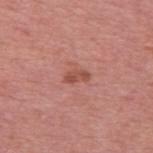biopsy status=imaged on a skin check; not biopsied
lesion diameter=≈2.5 mm
imaging modality=~15 mm tile from a whole-body skin photo
patient=male, approximately 55 years of age
anatomic site=the back
tile lighting=white-light illumination